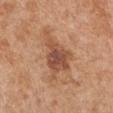The lesion was photographed on a routine skin check and not biopsied; there is no pathology result. The lesion-visualizer software estimated a border-irregularity index near 6.5/10, internal color variation of about 4.5 on a 0–10 scale, and a peripheral color-asymmetry measure near 1. The analysis additionally found a classifier nevus-likeness of about 15/100 and a detector confidence of about 100 out of 100 that the crop contains a lesion. The subject is a male roughly 65 years of age. This image is a 15 mm lesion crop taken from a total-body photograph. The lesion is on the left upper arm. Captured under white-light illumination. About 6.5 mm across.